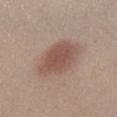No biopsy was performed on this lesion — it was imaged during a full skin examination and was not determined to be concerning. From the left lower leg. Longest diameter approximately 6 mm. A female patient, aged approximately 25. A roughly 15 mm field-of-view crop from a total-body skin photograph.